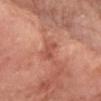Recorded during total-body skin imaging; not selected for excision or biopsy. This image is a 15 mm lesion crop taken from a total-body photograph. The lesion-visualizer software estimated a color-variation rating of about 0.5/10 and a peripheral color-asymmetry measure near 0. It also reported a nevus-likeness score of about 0/100 and lesion-presence confidence of about 100/100. Measured at roughly 3 mm in maximum diameter. On the chest. The tile uses white-light illumination. The patient is a female in their mid-70s.